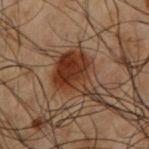Assessment: Recorded during total-body skin imaging; not selected for excision or biopsy. Background: Approximately 7 mm at its widest. An algorithmic analysis of the crop reported a border-irregularity rating of about 6/10, a within-lesion color-variation index near 4.5/10, and a peripheral color-asymmetry measure near 1.5. The software also gave a classifier nevus-likeness of about 100/100 and lesion-presence confidence of about 100/100. On the arm. A male patient aged 48–52. This image is a 15 mm lesion crop taken from a total-body photograph. Imaged with cross-polarized lighting.